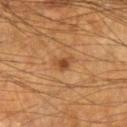Assessment:
The lesion was tiled from a total-body skin photograph and was not biopsied.
Background:
From the right forearm. The subject is a male in their mid-60s. A 15 mm close-up tile from a total-body photography series done for melanoma screening. Automated image analysis of the tile measured an automated nevus-likeness rating near 80 out of 100. Captured under cross-polarized illumination.